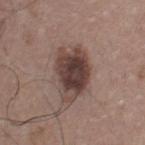<tbp_lesion>
  <biopsy_status>not biopsied; imaged during a skin examination</biopsy_status>
  <patient>
    <sex>male</sex>
    <age_approx>40</age_approx>
  </patient>
  <automated_metrics>
    <eccentricity>0.8</eccentricity>
    <shape_asymmetry>0.25</shape_asymmetry>
  </automated_metrics>
  <site>chest</site>
  <lesion_size>
    <long_diameter_mm_approx>6.5</long_diameter_mm_approx>
  </lesion_size>
  <image>
    <source>total-body photography crop</source>
    <field_of_view_mm>15</field_of_view_mm>
  </image>
</tbp_lesion>